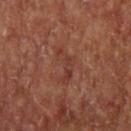No biopsy was performed on this lesion — it was imaged during a full skin examination and was not determined to be concerning. Imaged with cross-polarized lighting. From the upper back. A roughly 15 mm field-of-view crop from a total-body skin photograph. Automated tile analysis of the lesion measured an eccentricity of roughly 0.85 and two-axis asymmetry of about 0.65. The analysis additionally found a lesion–skin lightness drop of about 5 and a normalized lesion–skin contrast near 5. The analysis additionally found a nevus-likeness score of about 0/100 and a lesion-detection confidence of about 95/100. The lesion's longest dimension is about 4.5 mm. A male subject, aged 53–57.